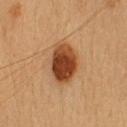Assessment: The lesion was tiled from a total-body skin photograph and was not biopsied. Context: A roughly 15 mm field-of-view crop from a total-body skin photograph. Captured under cross-polarized illumination. Automated tile analysis of the lesion measured an area of roughly 13 mm² and a shape-asymmetry score of about 0.15 (0 = symmetric). And it measured a lesion color around L≈38 a*≈23 b*≈33 in CIELAB, a lesion–skin lightness drop of about 16, and a normalized lesion–skin contrast near 12.5. And it measured a border-irregularity rating of about 1.5/10, internal color variation of about 5.5 on a 0–10 scale, and radial color variation of about 2. It also reported a nevus-likeness score of about 100/100 and a lesion-detection confidence of about 100/100. Longest diameter approximately 5 mm. On the head or neck. The subject is a female aged 18–22.